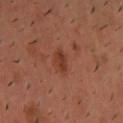tile lighting: cross-polarized; image source: ~15 mm crop, total-body skin-cancer survey; subject: male, roughly 40 years of age; body site: the head or neck.